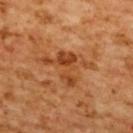Clinical impression: Part of a total-body skin-imaging series; this lesion was reviewed on a skin check and was not flagged for biopsy. Clinical summary: Imaged with cross-polarized lighting. The lesion is located on the upper back. A female subject, about 55 years old. A close-up tile cropped from a whole-body skin photograph, about 15 mm across.